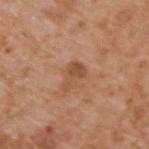Clinical impression:
Captured during whole-body skin photography for melanoma surveillance; the lesion was not biopsied.
Context:
A male subject roughly 65 years of age. This image is a 15 mm lesion crop taken from a total-body photograph. From the upper back. Imaged with white-light lighting.